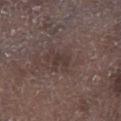workup = imaged on a skin check; not biopsied
acquisition = ~15 mm tile from a whole-body skin photo
lesion diameter = ≈2.5 mm
tile lighting = white-light
subject = male, approximately 70 years of age
anatomic site = the left lower leg